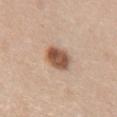{
  "site": "chest",
  "image": {
    "source": "total-body photography crop",
    "field_of_view_mm": 15
  },
  "lighting": "white-light",
  "patient": {
    "sex": "female",
    "age_approx": 30
  },
  "automated_metrics": {
    "eccentricity": 0.35,
    "shape_asymmetry": 0.15,
    "cielab_L": 54,
    "cielab_a": 20,
    "cielab_b": 30,
    "vs_skin_darker_L": 16.0,
    "vs_skin_contrast_norm": 10.5,
    "nevus_likeness_0_100": 100,
    "lesion_detection_confidence_0_100": 100
  }
}